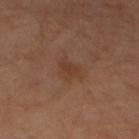biopsy status: imaged on a skin check; not biopsied | patient: male, aged around 30 | site: the left thigh | size: about 2.5 mm | image source: ~15 mm tile from a whole-body skin photo | automated metrics: a lesion area of about 4.5 mm², a shape eccentricity near 0.45, and a shape-asymmetry score of about 0.4 (0 = symmetric); a classifier nevus-likeness of about 0/100 and lesion-presence confidence of about 100/100.